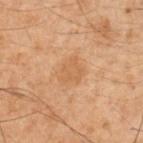* biopsy status — imaged on a skin check; not biopsied
* image — ~15 mm crop, total-body skin-cancer survey
* anatomic site — the right upper arm
* subject — male, aged around 50